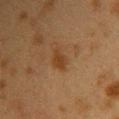Q: Is there a histopathology result?
A: total-body-photography surveillance lesion; no biopsy
Q: Where on the body is the lesion?
A: the upper back
Q: What lighting was used for the tile?
A: cross-polarized
Q: What is the lesion's diameter?
A: ~3 mm (longest diameter)
Q: What kind of image is this?
A: 15 mm crop, total-body photography
Q: Patient demographics?
A: female, roughly 40 years of age